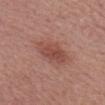{"biopsy_status": "not biopsied; imaged during a skin examination", "lighting": "white-light", "automated_metrics": {"eccentricity": 0.8, "shape_asymmetry": 0.2, "cielab_L": 48, "cielab_a": 24, "cielab_b": 26, "vs_skin_contrast_norm": 7.0, "nevus_likeness_0_100": 75}, "site": "head or neck", "image": {"source": "total-body photography crop", "field_of_view_mm": 15}, "patient": {"sex": "female", "age_approx": 50}}